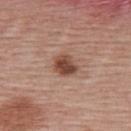Q: Was this lesion biopsied?
A: no biopsy performed (imaged during a skin exam)
Q: What are the patient's age and sex?
A: female, in their mid- to late 50s
Q: What kind of image is this?
A: ~15 mm crop, total-body skin-cancer survey
Q: What is the anatomic site?
A: the upper back